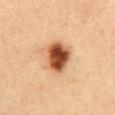Imaged during a routine full-body skin examination; the lesion was not biopsied and no histopathology is available.
The subject is a female approximately 40 years of age.
A roughly 15 mm field-of-view crop from a total-body skin photograph.
The lesion's longest dimension is about 4 mm.
The total-body-photography lesion software estimated a lesion color around L≈42 a*≈22 b*≈33 in CIELAB, a lesion–skin lightness drop of about 19, and a normalized border contrast of about 13.5.
Captured under cross-polarized illumination.
The lesion is on the abdomen.